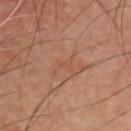Imaged during a routine full-body skin examination; the lesion was not biopsied and no histopathology is available. The tile uses cross-polarized illumination. A male patient, approximately 45 years of age. The lesion is located on the chest. Cropped from a whole-body photographic skin survey; the tile spans about 15 mm. Automated tile analysis of the lesion measured an area of roughly 1.5 mm², an outline eccentricity of about 0.85 (0 = round, 1 = elongated), and two-axis asymmetry of about 0.3. The software also gave a border-irregularity rating of about 3.5/10.